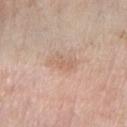Impression: Recorded during total-body skin imaging; not selected for excision or biopsy. Clinical summary: A 15 mm crop from a total-body photograph taken for skin-cancer surveillance. Longest diameter approximately 3 mm. Located on the right forearm. A female subject roughly 70 years of age. This is a white-light tile.